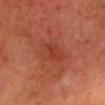lighting — cross-polarized | imaging modality — ~15 mm tile from a whole-body skin photo | location — the head or neck | size — ~2 mm (longest diameter) | automated lesion analysis — a lesion area of about 2 mm², a shape eccentricity near 0.85, and a symmetry-axis asymmetry near 0.4; a border-irregularity index near 4/10 and radial color variation of about 0 | patient — male, in their mid- to late 60s.